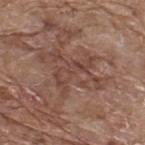<tbp_lesion>
  <biopsy_status>not biopsied; imaged during a skin examination</biopsy_status>
  <site>upper back</site>
  <lighting>white-light</lighting>
  <lesion_size>
    <long_diameter_mm_approx>5.0</long_diameter_mm_approx>
  </lesion_size>
  <automated_metrics>
    <vs_skin_darker_L>7.0</vs_skin_darker_L>
    <vs_skin_contrast_norm>5.5</vs_skin_contrast_norm>
    <peripheral_color_asymmetry>1.0</peripheral_color_asymmetry>
  </automated_metrics>
  <image>
    <source>total-body photography crop</source>
    <field_of_view_mm>15</field_of_view_mm>
  </image>
  <patient>
    <sex>male</sex>
    <age_approx>70</age_approx>
  </patient>
</tbp_lesion>